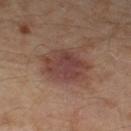biopsy status: total-body-photography surveillance lesion; no biopsy
imaging modality: total-body-photography crop, ~15 mm field of view
patient: male, in their mid-50s
tile lighting: cross-polarized illumination
automated lesion analysis: an area of roughly 16 mm², an outline eccentricity of about 0.7 (0 = round, 1 = elongated), and a symmetry-axis asymmetry near 0.2; an average lesion color of about L≈40 a*≈20 b*≈22 (CIELAB), about 9 CIELAB-L* units darker than the surrounding skin, and a normalized border contrast of about 8; a border-irregularity index near 2/10, internal color variation of about 3.5 on a 0–10 scale, and radial color variation of about 1
body site: the left thigh